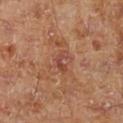follow-up: imaged on a skin check; not biopsied
lighting: cross-polarized illumination
subject: male, approximately 70 years of age
diameter: ≈3 mm
image source: 15 mm crop, total-body photography
location: the left lower leg
image-analysis metrics: a lesion area of about 3 mm² and an outline eccentricity of about 0.85 (0 = round, 1 = elongated); a lesion color around L≈44 a*≈25 b*≈28 in CIELAB, a lesion–skin lightness drop of about 8, and a normalized lesion–skin contrast near 6.5; border irregularity of about 6 on a 0–10 scale, a color-variation rating of about 0/10, and a peripheral color-asymmetry measure near 0